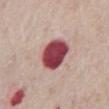{
  "biopsy_status": "not biopsied; imaged during a skin examination",
  "image": {
    "source": "total-body photography crop",
    "field_of_view_mm": 15
  },
  "patient": {
    "sex": "male",
    "age_approx": 75
  },
  "site": "front of the torso",
  "automated_metrics": {
    "area_mm2_approx": 13.0,
    "shape_asymmetry": 0.15,
    "border_irregularity_0_10": 1.5,
    "color_variation_0_10": 4.0,
    "nevus_likeness_0_100": 30,
    "lesion_detection_confidence_0_100": 100
  }
}